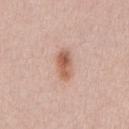Findings:
– lighting — white-light illumination
– subject — male, about 40 years old
– automated lesion analysis — a footprint of about 6.5 mm²
– size — about 3.5 mm
– image — 15 mm crop, total-body photography
– anatomic site — the chest